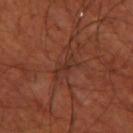Q: Was a biopsy performed?
A: catalogued during a skin exam; not biopsied
Q: What did automated image analysis measure?
A: a footprint of about 2.5 mm², an eccentricity of roughly 0.9, and a symmetry-axis asymmetry near 0.4; a border-irregularity rating of about 4.5/10, a color-variation rating of about 0/10, and peripheral color asymmetry of about 0
Q: Lesion location?
A: the leg
Q: What is the lesion's diameter?
A: ~2.5 mm (longest diameter)
Q: Who is the patient?
A: male, in their 70s
Q: What kind of image is this?
A: ~15 mm crop, total-body skin-cancer survey
Q: How was the tile lit?
A: cross-polarized illumination